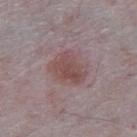{
  "biopsy_status": "not biopsied; imaged during a skin examination",
  "lesion_size": {
    "long_diameter_mm_approx": 4.0
  },
  "patient": {
    "sex": "male",
    "age_approx": 65
  },
  "image": {
    "source": "total-body photography crop",
    "field_of_view_mm": 15
  },
  "automated_metrics": {
    "eccentricity": 0.45,
    "shape_asymmetry": 0.2,
    "cielab_L": 48,
    "cielab_a": 19,
    "cielab_b": 18,
    "vs_skin_darker_L": 8.0,
    "vs_skin_contrast_norm": 7.0,
    "color_variation_0_10": 3.5
  },
  "lighting": "white-light",
  "site": "abdomen"
}